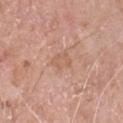Context:
Automated tile analysis of the lesion measured a lesion color around L≈60 a*≈21 b*≈30 in CIELAB, a lesion–skin lightness drop of about 6, and a normalized border contrast of about 4.5. Located on the front of the torso. The recorded lesion diameter is about 3 mm. The patient is a male in their mid- to late 60s. Imaged with white-light lighting. A lesion tile, about 15 mm wide, cut from a 3D total-body photograph.